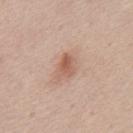The patient is a male aged 43 to 47.
The lesion's longest dimension is about 2.5 mm.
A 15 mm close-up extracted from a 3D total-body photography capture.
The tile uses white-light illumination.
From the mid back.
Automated image analysis of the tile measured an area of roughly 4.5 mm², an outline eccentricity of about 0.75 (0 = round, 1 = elongated), and a shape-asymmetry score of about 0.25 (0 = symmetric). The software also gave an average lesion color of about L≈58 a*≈21 b*≈29 (CIELAB) and a normalized lesion–skin contrast near 7.